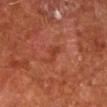Clinical impression:
The lesion was tiled from a total-body skin photograph and was not biopsied.
Image and clinical context:
A close-up tile cropped from a whole-body skin photograph, about 15 mm across. On the right lower leg. A male subject, aged 63–67. Approximately 2.5 mm at its widest.